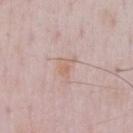The lesion was photographed on a routine skin check and not biopsied; there is no pathology result. The recorded lesion diameter is about 2.5 mm. Automated tile analysis of the lesion measured a footprint of about 3 mm² and two-axis asymmetry of about 0.5. The analysis additionally found a nevus-likeness score of about 15/100 and lesion-presence confidence of about 100/100. The patient is a male aged 48–52. Captured under white-light illumination. A roughly 15 mm field-of-view crop from a total-body skin photograph. On the chest.